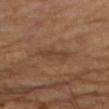patient: approximately 65 years of age | lighting: cross-polarized | location: the right upper arm | lesion diameter: ≈3.5 mm | image source: ~15 mm crop, total-body skin-cancer survey.